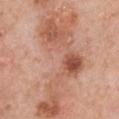This lesion was catalogued during total-body skin photography and was not selected for biopsy. This image is a 15 mm lesion crop taken from a total-body photograph. Imaged with white-light lighting. Automated tile analysis of the lesion measured an area of roughly 44 mm², an outline eccentricity of about 0.95 (0 = round, 1 = elongated), and two-axis asymmetry of about 0.65. And it measured a mean CIELAB color near L≈57 a*≈24 b*≈31, roughly 10 lightness units darker than nearby skin, and a normalized border contrast of about 7. And it measured a border-irregularity rating of about 10/10 and a peripheral color-asymmetry measure near 2.5. The analysis additionally found a nevus-likeness score of about 0/100 and a lesion-detection confidence of about 95/100. The subject is a female in their 60s. Located on the chest.